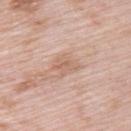Impression: Captured during whole-body skin photography for melanoma surveillance; the lesion was not biopsied. Image and clinical context: A female subject aged 63–67. A 15 mm close-up extracted from a 3D total-body photography capture. The lesion is on the back. The lesion's longest dimension is about 3 mm. Captured under white-light illumination.